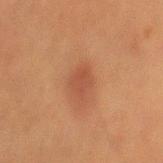follow-up=no biopsy performed (imaged during a skin exam) | diameter=about 3 mm | patient=male, roughly 50 years of age | TBP lesion metrics=a lesion area of about 5 mm² and two-axis asymmetry of about 0.25; a border-irregularity rating of about 2.5/10, a within-lesion color-variation index near 1.5/10, and a peripheral color-asymmetry measure near 0.5 | acquisition=15 mm crop, total-body photography | site=the mid back.